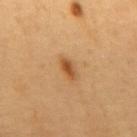Findings:
* biopsy status — catalogued during a skin exam; not biopsied
* location — the mid back
* acquisition — ~15 mm tile from a whole-body skin photo
* subject — male, approximately 60 years of age
* automated lesion analysis — a lesion color around L≈51 a*≈23 b*≈41 in CIELAB and a lesion-to-skin contrast of about 8.5 (normalized; higher = more distinct); a border-irregularity index near 2.5/10, a within-lesion color-variation index near 2.5/10, and peripheral color asymmetry of about 1; a classifier nevus-likeness of about 90/100 and a detector confidence of about 100 out of 100 that the crop contains a lesion
* diameter — about 3 mm